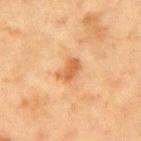  biopsy_status: not biopsied; imaged during a skin examination
  automated_metrics:
    area_mm2_approx: 5.0
    eccentricity: 0.75
    shape_asymmetry: 0.3
    cielab_L: 54
    cielab_a: 23
    cielab_b: 38
    vs_skin_darker_L: 10.0
    vs_skin_contrast_norm: 7.5
    color_variation_0_10: 1.5
    peripheral_color_asymmetry: 0.5
  image:
    source: total-body photography crop
    field_of_view_mm: 15
  patient:
    sex: female
    age_approx: 40
  lighting: cross-polarized
  lesion_size:
    long_diameter_mm_approx: 3.0
  site: arm